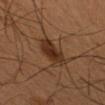Case summary:
– biopsy status · no biopsy performed (imaged during a skin exam)
– patient · female, approximately 50 years of age
– image source · 15 mm crop, total-body photography
– anatomic site · the left forearm